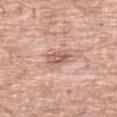No biopsy was performed on this lesion — it was imaged during a full skin examination and was not determined to be concerning.
A 15 mm close-up extracted from a 3D total-body photography capture.
The tile uses white-light illumination.
The subject is a male aged approximately 80.
The lesion-visualizer software estimated roughly 11 lightness units darker than nearby skin and a normalized border contrast of about 7. The analysis additionally found a border-irregularity rating of about 1.5/10, a within-lesion color-variation index near 4.5/10, and peripheral color asymmetry of about 2. The software also gave an automated nevus-likeness rating near 0 out of 100 and a lesion-detection confidence of about 100/100.
The lesion is on the left lower leg.
Approximately 3 mm at its widest.